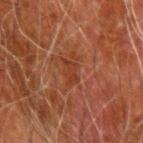Impression:
The lesion was photographed on a routine skin check and not biopsied; there is no pathology result.
Clinical summary:
On the right upper arm. A 15 mm crop from a total-body photograph taken for skin-cancer surveillance. The tile uses cross-polarized illumination. Longest diameter approximately 2.5 mm. The patient is a male in their 80s.